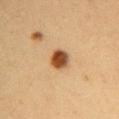No biopsy was performed on this lesion — it was imaged during a full skin examination and was not determined to be concerning.
Captured under cross-polarized illumination.
The subject is a female approximately 30 years of age.
A region of skin cropped from a whole-body photographic capture, roughly 15 mm wide.
Automated tile analysis of the lesion measured an automated nevus-likeness rating near 100 out of 100.
Approximately 2.5 mm at its widest.
On the right upper arm.